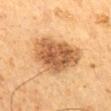notes: catalogued during a skin exam; not biopsied
image: total-body-photography crop, ~15 mm field of view
image-analysis metrics: a footprint of about 25 mm² and a shape eccentricity near 0.8; an automated nevus-likeness rating near 90 out of 100 and a lesion-detection confidence of about 100/100
lesion size: ≈7 mm
subject: male, aged 58 to 62
location: the back
illumination: cross-polarized